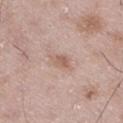Background: A male patient aged approximately 50. This is a white-light tile. A lesion tile, about 15 mm wide, cut from a 3D total-body photograph. Measured at roughly 2.5 mm in maximum diameter. The lesion is located on the right thigh.